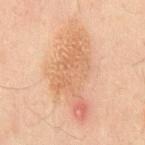Case summary:
– notes — imaged on a skin check; not biopsied
– patient — male, aged 48 to 52
– imaging modality — total-body-photography crop, ~15 mm field of view
– tile lighting — cross-polarized
– site — the mid back
– lesion size — ~11.5 mm (longest diameter)